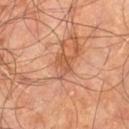The lesion was tiled from a total-body skin photograph and was not biopsied. The lesion-visualizer software estimated a lesion color around L≈53 a*≈24 b*≈35 in CIELAB, roughly 8 lightness units darker than nearby skin, and a normalized border contrast of about 6. The software also gave border irregularity of about 5 on a 0–10 scale, a color-variation rating of about 2/10, and peripheral color asymmetry of about 1. The software also gave a nevus-likeness score of about 0/100 and a lesion-detection confidence of about 100/100. A male patient about 60 years old. Cropped from a whole-body photographic skin survey; the tile spans about 15 mm. Located on the right leg. Captured under cross-polarized illumination. Measured at roughly 3 mm in maximum diameter.